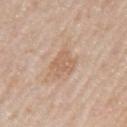{"biopsy_status": "not biopsied; imaged during a skin examination", "patient": {"sex": "male", "age_approx": 75}, "lesion_size": {"long_diameter_mm_approx": 3.5}, "site": "left upper arm", "automated_metrics": {"area_mm2_approx": 6.0, "shape_asymmetry": 0.35, "cielab_L": 62, "cielab_a": 19, "cielab_b": 31, "vs_skin_darker_L": 8.0, "vs_skin_contrast_norm": 5.5, "lesion_detection_confidence_0_100": 100}, "lighting": "white-light", "image": {"source": "total-body photography crop", "field_of_view_mm": 15}}